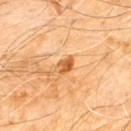<tbp_lesion>
  <biopsy_status>not biopsied; imaged during a skin examination</biopsy_status>
  <patient>
    <sex>male</sex>
    <age_approx>60</age_approx>
  </patient>
  <site>chest</site>
  <image>
    <source>total-body photography crop</source>
    <field_of_view_mm>15</field_of_view_mm>
  </image>
</tbp_lesion>